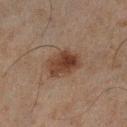The lesion was tiled from a total-body skin photograph and was not biopsied. A male subject in their mid-40s. The lesion-visualizer software estimated a shape eccentricity near 0.75 and a symmetry-axis asymmetry near 0.2. A 15 mm close-up extracted from a 3D total-body photography capture. Located on the left lower leg. Captured under cross-polarized illumination. Approximately 4.5 mm at its widest.